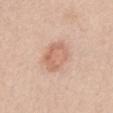Q: What kind of image is this?
A: ~15 mm crop, total-body skin-cancer survey
Q: What is the anatomic site?
A: the chest
Q: Illumination type?
A: white-light
Q: What did automated image analysis measure?
A: a lesion area of about 11 mm² and a symmetry-axis asymmetry near 0.1; a within-lesion color-variation index near 3.5/10
Q: Patient demographics?
A: female, about 40 years old